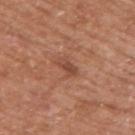{"site": "upper back", "image": {"source": "total-body photography crop", "field_of_view_mm": 15}, "patient": {"sex": "male", "age_approx": 65}, "lighting": "white-light"}